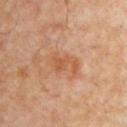Captured during whole-body skin photography for melanoma surveillance; the lesion was not biopsied.
The patient is a male aged 53 to 57.
From the chest.
The lesion's longest dimension is about 3.5 mm.
A roughly 15 mm field-of-view crop from a total-body skin photograph.
The tile uses cross-polarized illumination.